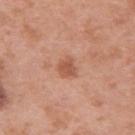Recorded during total-body skin imaging; not selected for excision or biopsy.
The lesion is located on the arm.
A female subject aged 38–42.
A region of skin cropped from a whole-body photographic capture, roughly 15 mm wide.
Automated tile analysis of the lesion measured an area of roughly 4.5 mm² and a shape eccentricity near 0.6. It also reported an average lesion color of about L≈55 a*≈26 b*≈32 (CIELAB), about 10 CIELAB-L* units darker than the surrounding skin, and a normalized lesion–skin contrast near 6.5. The analysis additionally found border irregularity of about 3 on a 0–10 scale, a within-lesion color-variation index near 2/10, and peripheral color asymmetry of about 0.5.
This is a white-light tile.
Measured at roughly 2.5 mm in maximum diameter.